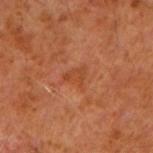notes: catalogued during a skin exam; not biopsied
patient: male, about 60 years old
acquisition: 15 mm crop, total-body photography
tile lighting: cross-polarized
body site: the right upper arm
lesion diameter: ~2.5 mm (longest diameter)
automated metrics: border irregularity of about 5.5 on a 0–10 scale, a within-lesion color-variation index near 1.5/10, and peripheral color asymmetry of about 0.5; an automated nevus-likeness rating near 0 out of 100 and lesion-presence confidence of about 100/100